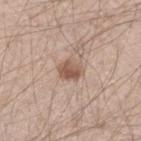Case summary:
– biopsy status — catalogued during a skin exam; not biopsied
– subject — male, aged around 30
– diameter — ~3 mm (longest diameter)
– anatomic site — the arm
– image source — total-body-photography crop, ~15 mm field of view
– tile lighting — white-light illumination